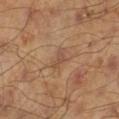The lesion was photographed on a routine skin check and not biopsied; there is no pathology result. A 15 mm close-up tile from a total-body photography series done for melanoma screening. Located on the leg. Measured at roughly 2.5 mm in maximum diameter. This is a cross-polarized tile. A male patient aged around 45.